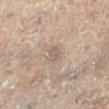biopsy status: no biopsy performed (imaged during a skin exam)
size: ≈2.5 mm
automated metrics: a footprint of about 3.5 mm², an outline eccentricity of about 0.8 (0 = round, 1 = elongated), and a shape-asymmetry score of about 0.25 (0 = symmetric); a classifier nevus-likeness of about 0/100 and lesion-presence confidence of about 90/100
subject: female, aged approximately 80
anatomic site: the leg
lighting: cross-polarized
image: ~15 mm crop, total-body skin-cancer survey